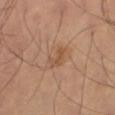Notes:
• notes — catalogued during a skin exam; not biopsied
• lesion diameter — ~2.5 mm (longest diameter)
• automated lesion analysis — a lesion area of about 3 mm² and an eccentricity of roughly 0.85; an average lesion color of about L≈52 a*≈21 b*≈34 (CIELAB), about 7 CIELAB-L* units darker than the surrounding skin, and a lesion-to-skin contrast of about 5.5 (normalized; higher = more distinct)
• lighting — cross-polarized illumination
• body site — the right thigh
• image source — total-body-photography crop, ~15 mm field of view